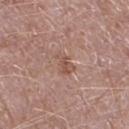notes: imaged on a skin check; not biopsied
lighting: white-light illumination
location: the left lower leg
subject: male, about 50 years old
diameter: ~3 mm (longest diameter)
acquisition: ~15 mm crop, total-body skin-cancer survey
image-analysis metrics: a mean CIELAB color near L≈52 a*≈21 b*≈26, about 8 CIELAB-L* units darker than the surrounding skin, and a normalized lesion–skin contrast near 6